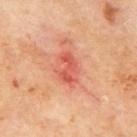Case summary:
– illumination — cross-polarized
– subject — male, aged 68 to 72
– diameter — ~3.5 mm (longest diameter)
– location — the mid back
– imaging modality — 15 mm crop, total-body photography